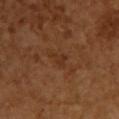Imaged during a routine full-body skin examination; the lesion was not biopsied and no histopathology is available.
The total-body-photography lesion software estimated a symmetry-axis asymmetry near 0.4. The analysis additionally found a lesion color around L≈32 a*≈22 b*≈31 in CIELAB, a lesion–skin lightness drop of about 5, and a normalized lesion–skin contrast near 5. It also reported a border-irregularity index near 4.5/10, internal color variation of about 0.5 on a 0–10 scale, and a peripheral color-asymmetry measure near 0.
From the chest.
A female patient in their mid-50s.
Cropped from a whole-body photographic skin survey; the tile spans about 15 mm.